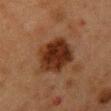Recorded during total-body skin imaging; not selected for excision or biopsy. From the chest. Cropped from a total-body skin-imaging series; the visible field is about 15 mm. The total-body-photography lesion software estimated an area of roughly 17 mm², a shape eccentricity near 0.5, and a symmetry-axis asymmetry near 0.15. The subject is a female aged around 50.